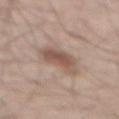This lesion was catalogued during total-body skin photography and was not selected for biopsy. Captured under white-light illumination. The lesion is on the mid back. An algorithmic analysis of the crop reported an eccentricity of roughly 0.95. And it measured a mean CIELAB color near L≈54 a*≈17 b*≈25, roughly 11 lightness units darker than nearby skin, and a normalized lesion–skin contrast near 7.5. The analysis additionally found a nevus-likeness score of about 90/100 and lesion-presence confidence of about 100/100. About 6 mm across. The patient is a male aged around 50. A region of skin cropped from a whole-body photographic capture, roughly 15 mm wide.